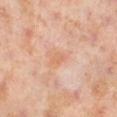Imaged during a routine full-body skin examination; the lesion was not biopsied and no histopathology is available. A female subject aged around 50. Automated image analysis of the tile measured a shape eccentricity near 0.85 and two-axis asymmetry of about 0.3. From the left thigh. The recorded lesion diameter is about 2.5 mm. A 15 mm crop from a total-body photograph taken for skin-cancer surveillance.